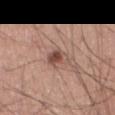Part of a total-body skin-imaging series; this lesion was reviewed on a skin check and was not flagged for biopsy. About 3 mm across. The lesion is located on the left thigh. A male patient, aged approximately 60. A close-up tile cropped from a whole-body skin photograph, about 15 mm across. The tile uses white-light illumination. Automated image analysis of the tile measured an average lesion color of about L≈50 a*≈20 b*≈25 (CIELAB), a lesion–skin lightness drop of about 11, and a normalized border contrast of about 8. The analysis additionally found border irregularity of about 3 on a 0–10 scale, a within-lesion color-variation index near 6/10, and peripheral color asymmetry of about 2.